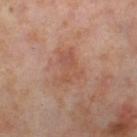Part of a total-body skin-imaging series; this lesion was reviewed on a skin check and was not flagged for biopsy.
This is a cross-polarized tile.
Approximately 4 mm at its widest.
The subject is a female aged approximately 55.
A roughly 15 mm field-of-view crop from a total-body skin photograph.
The lesion is on the right thigh.